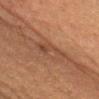Clinical impression: Recorded during total-body skin imaging; not selected for excision or biopsy. Background: The total-body-photography lesion software estimated a lesion area of about 5.5 mm². It also reported a lesion color around L≈36 a*≈18 b*≈26 in CIELAB, about 6 CIELAB-L* units darker than the surrounding skin, and a normalized lesion–skin contrast near 6. It also reported a classifier nevus-likeness of about 0/100. The tile uses cross-polarized illumination. A female subject, in their mid- to late 50s. This image is a 15 mm lesion crop taken from a total-body photograph. The lesion is on the head or neck.